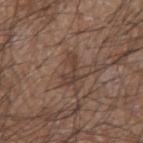Part of a total-body skin-imaging series; this lesion was reviewed on a skin check and was not flagged for biopsy. A male subject aged 53–57. The tile uses white-light illumination. A lesion tile, about 15 mm wide, cut from a 3D total-body photograph. On the left forearm.